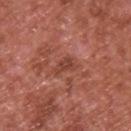Clinical impression: This lesion was catalogued during total-body skin photography and was not selected for biopsy. Context: The lesion's longest dimension is about 3 mm. The patient is a male roughly 65 years of age. Automated tile analysis of the lesion measured a lesion area of about 4 mm² and an eccentricity of roughly 0.85. The software also gave an average lesion color of about L≈43 a*≈27 b*≈28 (CIELAB), a lesion–skin lightness drop of about 8, and a lesion-to-skin contrast of about 6 (normalized; higher = more distinct). The software also gave a within-lesion color-variation index near 2.5/10 and peripheral color asymmetry of about 1. From the upper back. Captured under white-light illumination. A close-up tile cropped from a whole-body skin photograph, about 15 mm across.